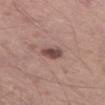  biopsy_status: not biopsied; imaged during a skin examination
  patient:
    sex: male
    age_approx: 50
  site: left thigh
  image:
    source: total-body photography crop
    field_of_view_mm: 15
  lesion_size:
    long_diameter_mm_approx: 3.0
  automated_metrics:
    area_mm2_approx: 4.5
    eccentricity: 0.65
    shape_asymmetry: 0.2
    peripheral_color_asymmetry: 1.5